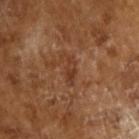Findings:
– workup · imaged on a skin check; not biopsied